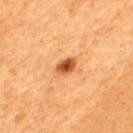Part of a total-body skin-imaging series; this lesion was reviewed on a skin check and was not flagged for biopsy. Approximately 3 mm at its widest. On the back. A 15 mm close-up extracted from a 3D total-body photography capture. Automated tile analysis of the lesion measured a mean CIELAB color near L≈48 a*≈27 b*≈39, a lesion–skin lightness drop of about 15, and a normalized border contrast of about 10. This is a cross-polarized tile. A male patient, about 65 years old.